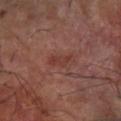No biopsy was performed on this lesion — it was imaged during a full skin examination and was not determined to be concerning. The recorded lesion diameter is about 3 mm. A male patient roughly 65 years of age. A 15 mm crop from a total-body photograph taken for skin-cancer surveillance. The lesion is located on the right forearm.